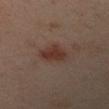biopsy status: total-body-photography surveillance lesion; no biopsy | site: the left forearm | image source: ~15 mm crop, total-body skin-cancer survey | subject: female, aged approximately 40 | automated metrics: a lesion area of about 7.5 mm², a shape eccentricity near 0.65, and a shape-asymmetry score of about 0.2 (0 = symmetric) | lesion size: ≈3.5 mm.